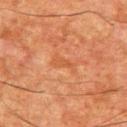Imaged during a routine full-body skin examination; the lesion was not biopsied and no histopathology is available.
The subject is a male about 65 years old.
A 15 mm crop from a total-body photograph taken for skin-cancer surveillance.
The lesion is on the upper back.